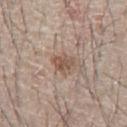The lesion was photographed on a routine skin check and not biopsied; there is no pathology result. A male subject in their mid- to late 60s. The tile uses white-light illumination. The lesion's longest dimension is about 3 mm. The lesion is located on the chest. This image is a 15 mm lesion crop taken from a total-body photograph.